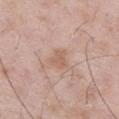{"biopsy_status": "not biopsied; imaged during a skin examination", "automated_metrics": {"eccentricity": 0.5, "shape_asymmetry": 0.35, "lesion_detection_confidence_0_100": 100}, "lighting": "white-light", "image": {"source": "total-body photography crop", "field_of_view_mm": 15}, "lesion_size": {"long_diameter_mm_approx": 2.5}, "site": "right thigh", "patient": {"sex": "male", "age_approx": 50}}